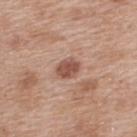No biopsy was performed on this lesion — it was imaged during a full skin examination and was not determined to be concerning. Captured under white-light illumination. A 15 mm close-up extracted from a 3D total-body photography capture. The lesion is located on the upper back. A female subject aged approximately 40. About 3 mm across. Automated tile analysis of the lesion measured an area of roughly 5 mm² and a symmetry-axis asymmetry near 0.2. And it measured a border-irregularity rating of about 2/10, internal color variation of about 2 on a 0–10 scale, and a peripheral color-asymmetry measure near 0.5.